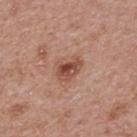The lesion was photographed on a routine skin check and not biopsied; there is no pathology result.
A 15 mm crop from a total-body photograph taken for skin-cancer surveillance.
This is a white-light tile.
From the upper back.
Longest diameter approximately 3.5 mm.
A female subject, approximately 65 years of age.